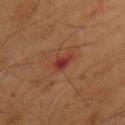No biopsy was performed on this lesion — it was imaged during a full skin examination and was not determined to be concerning. Captured under cross-polarized illumination. From the chest. Automated image analysis of the tile measured a footprint of about 3.5 mm², a shape eccentricity near 0.85, and a shape-asymmetry score of about 0.35 (0 = symmetric). It also reported a lesion color around L≈36 a*≈29 b*≈28 in CIELAB, roughly 9 lightness units darker than nearby skin, and a normalized border contrast of about 8.5. And it measured internal color variation of about 2 on a 0–10 scale. Cropped from a total-body skin-imaging series; the visible field is about 15 mm. A male patient aged around 55. The recorded lesion diameter is about 2.5 mm.